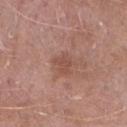Clinical impression:
Imaged during a routine full-body skin examination; the lesion was not biopsied and no histopathology is available.
Background:
This is a white-light tile. A male patient, aged approximately 55. Located on the right forearm. A 15 mm crop from a total-body photograph taken for skin-cancer surveillance.